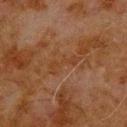Captured during whole-body skin photography for melanoma surveillance; the lesion was not biopsied.
An algorithmic analysis of the crop reported a lesion area of about 4 mm², a shape eccentricity near 0.95, and a symmetry-axis asymmetry near 0.45. The software also gave internal color variation of about 0 on a 0–10 scale and radial color variation of about 0.
Located on the upper back.
This image is a 15 mm lesion crop taken from a total-body photograph.
Captured under cross-polarized illumination.
The patient is a male about 80 years old.